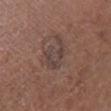{
  "site": "leg",
  "automated_metrics": {
    "eccentricity": 0.75,
    "shape_asymmetry": 0.55,
    "vs_skin_darker_L": 6.0,
    "vs_skin_contrast_norm": 6.0,
    "border_irregularity_0_10": 7.0
  },
  "lesion_size": {
    "long_diameter_mm_approx": 4.0
  },
  "patient": {
    "sex": "female",
    "age_approx": 50
  },
  "image": {
    "source": "total-body photography crop",
    "field_of_view_mm": 15
  },
  "lighting": "white-light"
}